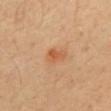The lesion was photographed on a routine skin check and not biopsied; there is no pathology result.
Located on the mid back.
Measured at roughly 3 mm in maximum diameter.
This image is a 15 mm lesion crop taken from a total-body photograph.
A male patient in their 30s.
This is a cross-polarized tile.